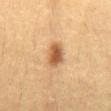{
  "biopsy_status": "not biopsied; imaged during a skin examination",
  "image": {
    "source": "total-body photography crop",
    "field_of_view_mm": 15
  },
  "site": "front of the torso",
  "patient": {
    "sex": "female",
    "age_approx": 30
  }
}